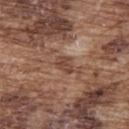Assessment:
Recorded during total-body skin imaging; not selected for excision or biopsy.
Image and clinical context:
The subject is a male in their mid-70s. Longest diameter approximately 2.5 mm. The lesion-visualizer software estimated a border-irregularity index near 2.5/10, internal color variation of about 2.5 on a 0–10 scale, and a peripheral color-asymmetry measure near 1. It also reported a classifier nevus-likeness of about 0/100 and a detector confidence of about 100 out of 100 that the crop contains a lesion. From the back. A lesion tile, about 15 mm wide, cut from a 3D total-body photograph.